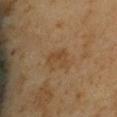biopsy_status: not biopsied; imaged during a skin examination
lesion_size:
  long_diameter_mm_approx: 3.0
image:
  source: total-body photography crop
  field_of_view_mm: 15
lighting: cross-polarized
patient:
  sex: male
  age_approx: 65